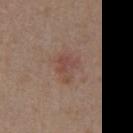Findings:
– workup — total-body-photography surveillance lesion; no biopsy
– acquisition — total-body-photography crop, ~15 mm field of view
– image-analysis metrics — lesion-presence confidence of about 100/100
– tile lighting — white-light illumination
– lesion diameter — about 3.5 mm
– body site — the right upper arm
– patient — male, aged 53 to 57